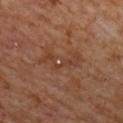| feature | finding |
|---|---|
| follow-up | total-body-photography surveillance lesion; no biopsy |
| anatomic site | the upper back |
| tile lighting | cross-polarized |
| subject | female, approximately 60 years of age |
| image-analysis metrics | a footprint of about 7 mm² and two-axis asymmetry of about 0.65; a mean CIELAB color near L≈34 a*≈19 b*≈26 and a lesion-to-skin contrast of about 5 (normalized; higher = more distinct); a border-irregularity index near 10/10, a color-variation rating of about 2/10, and a peripheral color-asymmetry measure near 0.5 |
| image | 15 mm crop, total-body photography |
| lesion diameter | about 5 mm |